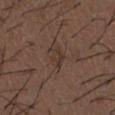biopsy_status: not biopsied; imaged during a skin examination
site: front of the torso
lesion_size:
  long_diameter_mm_approx: 2.5
image:
  source: total-body photography crop
  field_of_view_mm: 15
patient:
  sex: male
  age_approx: 50
lighting: white-light